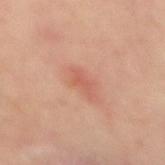The lesion was tiled from a total-body skin photograph and was not biopsied.
Cropped from a total-body skin-imaging series; the visible field is about 15 mm.
Located on the mid back.
An algorithmic analysis of the crop reported a mean CIELAB color near L≈57 a*≈27 b*≈30 and a normalized lesion–skin contrast near 5. And it measured a nevus-likeness score of about 5/100 and a lesion-detection confidence of about 100/100.
A male patient aged 48 to 52.
The tile uses cross-polarized illumination.
About 3 mm across.